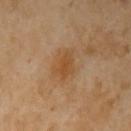Case summary:
- follow-up: no biopsy performed (imaged during a skin exam)
- image: total-body-photography crop, ~15 mm field of view
- patient: female, aged 68 to 72
- diameter: ~4.5 mm (longest diameter)
- anatomic site: the arm
- image-analysis metrics: border irregularity of about 3 on a 0–10 scale, a color-variation rating of about 3.5/10, and a peripheral color-asymmetry measure near 1; a classifier nevus-likeness of about 45/100 and lesion-presence confidence of about 100/100